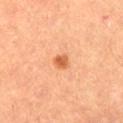The lesion was photographed on a routine skin check and not biopsied; there is no pathology result.
On the left thigh.
A lesion tile, about 15 mm wide, cut from a 3D total-body photograph.
A male subject in their mid- to late 60s.